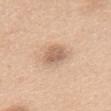Captured during whole-body skin photography for melanoma surveillance; the lesion was not biopsied.
The total-body-photography lesion software estimated a lesion color around L≈62 a*≈18 b*≈30 in CIELAB and a normalized lesion–skin contrast near 7.
About 3 mm across.
A female subject, in their mid-50s.
From the chest.
A 15 mm crop from a total-body photograph taken for skin-cancer surveillance.
This is a white-light tile.